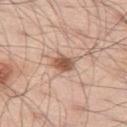biopsy status — imaged on a skin check; not biopsied | lesion diameter — ≈3 mm | imaging modality — ~15 mm crop, total-body skin-cancer survey | image-analysis metrics — a mean CIELAB color near L≈56 a*≈21 b*≈30, roughly 14 lightness units darker than nearby skin, and a normalized lesion–skin contrast near 9.5; border irregularity of about 1.5 on a 0–10 scale and a within-lesion color-variation index near 3.5/10; a detector confidence of about 100 out of 100 that the crop contains a lesion | patient — male, aged 53–57 | body site — the left thigh.